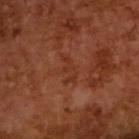The lesion was tiled from a total-body skin photograph and was not biopsied. A male subject about 65 years old. Measured at roughly 3.5 mm in maximum diameter. The lesion-visualizer software estimated an area of roughly 4 mm², an outline eccentricity of about 0.85 (0 = round, 1 = elongated), and a shape-asymmetry score of about 0.45 (0 = symmetric). It also reported a lesion color around L≈33 a*≈25 b*≈31 in CIELAB, a lesion–skin lightness drop of about 5, and a normalized lesion–skin contrast near 5. It also reported a border-irregularity rating of about 6/10 and a peripheral color-asymmetry measure near 0. Cropped from a total-body skin-imaging series; the visible field is about 15 mm.